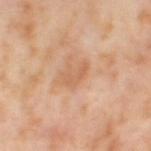<lesion>
  <biopsy_status>not biopsied; imaged during a skin examination</biopsy_status>
  <patient>
    <sex>female</sex>
    <age_approx>55</age_approx>
  </patient>
  <site>left thigh</site>
  <image>
    <source>total-body photography crop</source>
    <field_of_view_mm>15</field_of_view_mm>
  </image>
</lesion>